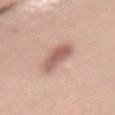<record>
  <biopsy_status>not biopsied; imaged during a skin examination</biopsy_status>
  <automated_metrics>
    <cielab_L>59</cielab_L>
    <cielab_a>21</cielab_a>
    <cielab_b>25</cielab_b>
    <vs_skin_darker_L>13.0</vs_skin_darker_L>
    <vs_skin_contrast_norm>8.0</vs_skin_contrast_norm>
    <border_irregularity_0_10>4.0</border_irregularity_0_10>
    <color_variation_0_10>2.5</color_variation_0_10>
    <nevus_likeness_0_100>65</nevus_likeness_0_100>
    <lesion_detection_confidence_0_100>100</lesion_detection_confidence_0_100>
  </automated_metrics>
  <site>abdomen</site>
  <lesion_size>
    <long_diameter_mm_approx>6.0</long_diameter_mm_approx>
  </lesion_size>
  <image>
    <source>total-body photography crop</source>
    <field_of_view_mm>15</field_of_view_mm>
  </image>
  <patient>
    <sex>female</sex>
    <age_approx>35</age_approx>
  </patient>
  <lighting>white-light</lighting>
</record>